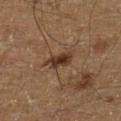Q: Is there a histopathology result?
A: total-body-photography surveillance lesion; no biopsy
Q: What kind of image is this?
A: 15 mm crop, total-body photography
Q: Automated lesion metrics?
A: a mean CIELAB color near L≈27 a*≈15 b*≈24, roughly 10 lightness units darker than nearby skin, and a normalized lesion–skin contrast near 10.5; a classifier nevus-likeness of about 85/100 and a detector confidence of about 100 out of 100 that the crop contains a lesion
Q: How was the tile lit?
A: cross-polarized illumination
Q: How large is the lesion?
A: ≈3 mm
Q: Where on the body is the lesion?
A: the left lower leg
Q: Who is the patient?
A: male, in their mid- to late 60s